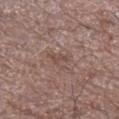Captured during whole-body skin photography for melanoma surveillance; the lesion was not biopsied. From the leg. This is a white-light tile. A region of skin cropped from a whole-body photographic capture, roughly 15 mm wide. Longest diameter approximately 3 mm. The lesion-visualizer software estimated a footprint of about 4 mm², an outline eccentricity of about 0.75 (0 = round, 1 = elongated), and two-axis asymmetry of about 0.45. And it measured a mean CIELAB color near L≈48 a*≈17 b*≈22, roughly 6 lightness units darker than nearby skin, and a normalized border contrast of about 5. A male subject roughly 70 years of age.